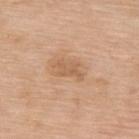<case>
  <lighting>white-light</lighting>
  <image>
    <source>total-body photography crop</source>
    <field_of_view_mm>15</field_of_view_mm>
  </image>
  <patient>
    <sex>female</sex>
    <age_approx>75</age_approx>
  </patient>
  <automated_metrics>
    <cielab_L>59</cielab_L>
    <cielab_a>20</cielab_a>
    <cielab_b>35</cielab_b>
    <vs_skin_darker_L>8.0</vs_skin_darker_L>
    <vs_skin_contrast_norm>5.5</vs_skin_contrast_norm>
  </automated_metrics>
  <lesion_size>
    <long_diameter_mm_approx>3.0</long_diameter_mm_approx>
  </lesion_size>
  <site>upper back</site>
</case>